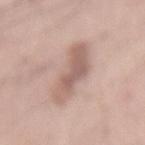Clinical impression:
The lesion was tiled from a total-body skin photograph and was not biopsied.
Background:
This image is a 15 mm lesion crop taken from a total-body photograph. The subject is a male aged 68 to 72. The total-body-photography lesion software estimated a nevus-likeness score of about 0/100. On the mid back. The tile uses white-light illumination.